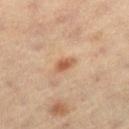Q: Was this lesion biopsied?
A: total-body-photography surveillance lesion; no biopsy
Q: What kind of image is this?
A: 15 mm crop, total-body photography
Q: What did automated image analysis measure?
A: a lesion area of about 3 mm² and two-axis asymmetry of about 0.25; a lesion–skin lightness drop of about 11 and a normalized border contrast of about 8; a border-irregularity rating of about 2.5/10, a within-lesion color-variation index near 1/10, and radial color variation of about 0.5
Q: What is the anatomic site?
A: the left thigh
Q: What lighting was used for the tile?
A: cross-polarized
Q: What is the lesion's diameter?
A: ~2.5 mm (longest diameter)
Q: Patient demographics?
A: male, aged around 60